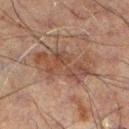Recorded during total-body skin imaging; not selected for excision or biopsy. Longest diameter approximately 7 mm. On the left lower leg. A male subject about 60 years old. A lesion tile, about 15 mm wide, cut from a 3D total-body photograph.